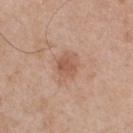Part of a total-body skin-imaging series; this lesion was reviewed on a skin check and was not flagged for biopsy.
The subject is a male aged 53 to 57.
Automated tile analysis of the lesion measured an automated nevus-likeness rating near 35 out of 100.
From the upper back.
A region of skin cropped from a whole-body photographic capture, roughly 15 mm wide.
The lesion's longest dimension is about 2.5 mm.